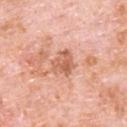Findings:
• biopsy status: total-body-photography surveillance lesion; no biopsy
• lesion diameter: ~3 mm (longest diameter)
• subject: male, about 80 years old
• acquisition: 15 mm crop, total-body photography
• site: the back
• lighting: white-light